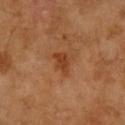Context:
The lesion-visualizer software estimated a lesion area of about 4 mm² and an eccentricity of roughly 0.75. The analysis additionally found about 9 CIELAB-L* units darker than the surrounding skin. The analysis additionally found a classifier nevus-likeness of about 65/100 and a detector confidence of about 100 out of 100 that the crop contains a lesion. Longest diameter approximately 3 mm. A 15 mm crop from a total-body photograph taken for skin-cancer surveillance. The tile uses cross-polarized illumination. A female subject aged approximately 70. The lesion is located on the right forearm.